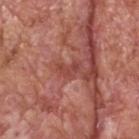No biopsy was performed on this lesion — it was imaged during a full skin examination and was not determined to be concerning. From the head or neck. The tile uses white-light illumination. The patient is a male about 60 years old. A lesion tile, about 15 mm wide, cut from a 3D total-body photograph.